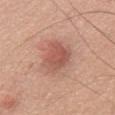workup: imaged on a skin check; not biopsied | anatomic site: the chest | illumination: white-light illumination | TBP lesion metrics: a footprint of about 10 mm² and an eccentricity of roughly 0.65; a mean CIELAB color near L≈55 a*≈25 b*≈27, a lesion–skin lightness drop of about 10, and a normalized lesion–skin contrast near 7; a border-irregularity index near 3/10, a within-lesion color-variation index near 3.5/10, and peripheral color asymmetry of about 1; a nevus-likeness score of about 75/100 | patient: male, roughly 75 years of age | diameter: ≈4 mm | imaging modality: ~15 mm tile from a whole-body skin photo.